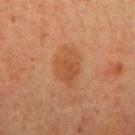No biopsy was performed on this lesion — it was imaged during a full skin examination and was not determined to be concerning.
Captured under cross-polarized illumination.
On the right upper arm.
The lesion's longest dimension is about 4.5 mm.
Automated tile analysis of the lesion measured a footprint of about 10 mm², an outline eccentricity of about 0.7 (0 = round, 1 = elongated), and a symmetry-axis asymmetry near 0.2. It also reported a mean CIELAB color near L≈41 a*≈21 b*≈31, about 6 CIELAB-L* units darker than the surrounding skin, and a normalized border contrast of about 5.5.
The patient is a female roughly 55 years of age.
A lesion tile, about 15 mm wide, cut from a 3D total-body photograph.